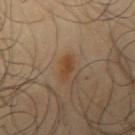Imaged during a routine full-body skin examination; the lesion was not biopsied and no histopathology is available. From the right upper arm. A male patient roughly 65 years of age. Cropped from a whole-body photographic skin survey; the tile spans about 15 mm. Imaged with cross-polarized lighting.